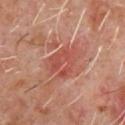  biopsy_status: not biopsied; imaged during a skin examination
  image:
    source: total-body photography crop
    field_of_view_mm: 15
  lighting: cross-polarized
  patient:
    sex: male
    age_approx: 60
  site: chest
  lesion_size:
    long_diameter_mm_approx: 4.0